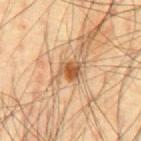Clinical impression:
Part of a total-body skin-imaging series; this lesion was reviewed on a skin check and was not flagged for biopsy.
Image and clinical context:
Automated tile analysis of the lesion measured an area of roughly 6 mm², a shape eccentricity near 0.7, and a shape-asymmetry score of about 0.25 (0 = symmetric). It also reported a lesion color around L≈55 a*≈20 b*≈36 in CIELAB, a lesion–skin lightness drop of about 12, and a normalized border contrast of about 8. The analysis additionally found an automated nevus-likeness rating near 90 out of 100 and a detector confidence of about 100 out of 100 that the crop contains a lesion. Captured under cross-polarized illumination. Measured at roughly 3.5 mm in maximum diameter. A male subject, aged approximately 45. The lesion is on the chest. A 15 mm crop from a total-body photograph taken for skin-cancer surveillance.